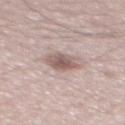notes — total-body-photography surveillance lesion; no biopsy | lighting — white-light | site — the mid back | lesion size — ≈3.5 mm | patient — male, approximately 50 years of age | imaging modality — total-body-photography crop, ~15 mm field of view.